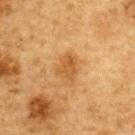Notes:
* biopsy status · imaged on a skin check; not biopsied
* subject · male, about 85 years old or older
* acquisition · total-body-photography crop, ~15 mm field of view
* anatomic site · the upper back